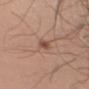No biopsy was performed on this lesion — it was imaged during a full skin examination and was not determined to be concerning. Longest diameter approximately 3 mm. A roughly 15 mm field-of-view crop from a total-body skin photograph. A male subject about 45 years old. On the left upper arm. Imaged with white-light lighting.